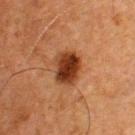Imaged during a routine full-body skin examination; the lesion was not biopsied and no histopathology is available.
The lesion's longest dimension is about 3.5 mm.
A lesion tile, about 15 mm wide, cut from a 3D total-body photograph.
A male subject about 50 years old.
This is a cross-polarized tile.
On the upper back.